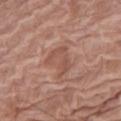<case>
  <biopsy_status>not biopsied; imaged during a skin examination</biopsy_status>
  <lesion_size>
    <long_diameter_mm_approx>3.5</long_diameter_mm_approx>
  </lesion_size>
  <site>right thigh</site>
  <lighting>white-light</lighting>
  <image>
    <source>total-body photography crop</source>
    <field_of_view_mm>15</field_of_view_mm>
  </image>
  <patient>
    <sex>female</sex>
    <age_approx>75</age_approx>
  </patient>
</case>